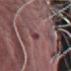Impression:
The lesion was tiled from a total-body skin photograph and was not biopsied.
Clinical summary:
The subject is a male approximately 75 years of age. The lesion is located on the leg. A lesion tile, about 15 mm wide, cut from a 3D total-body photograph.